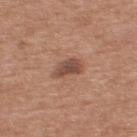A roughly 15 mm field-of-view crop from a total-body skin photograph.
An algorithmic analysis of the crop reported an average lesion color of about L≈48 a*≈21 b*≈27 (CIELAB). And it measured a border-irregularity index near 3/10, internal color variation of about 3 on a 0–10 scale, and a peripheral color-asymmetry measure near 1. The analysis additionally found a nevus-likeness score of about 65/100 and a lesion-detection confidence of about 100/100.
This is a white-light tile.
About 3.5 mm across.
From the upper back.
A male patient approximately 55 years of age.